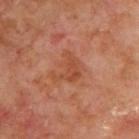follow-up: catalogued during a skin exam; not biopsied | image: ~15 mm crop, total-body skin-cancer survey | site: the back | diameter: about 3.5 mm | patient: male, about 70 years old | tile lighting: cross-polarized.